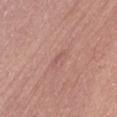This lesion was catalogued during total-body skin photography and was not selected for biopsy.
The patient is a male aged 38–42.
This is a white-light tile.
A close-up tile cropped from a whole-body skin photograph, about 15 mm across.
Longest diameter approximately 2.5 mm.
Located on the front of the torso.